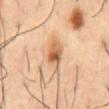Case summary:
* diameter · ≈4.5 mm
* automated metrics · an outline eccentricity of about 0.85 (0 = round, 1 = elongated) and two-axis asymmetry of about 0.2; an average lesion color of about L≈61 a*≈20 b*≈37 (CIELAB), roughly 12 lightness units darker than nearby skin, and a normalized lesion–skin contrast near 8; a nevus-likeness score of about 35/100 and a detector confidence of about 100 out of 100 that the crop contains a lesion
* illumination · cross-polarized
* anatomic site · the abdomen
* acquisition · 15 mm crop, total-body photography
* subject · male, in their mid-50s